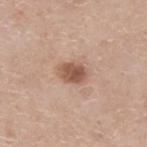{"biopsy_status": "not biopsied; imaged during a skin examination", "image": {"source": "total-body photography crop", "field_of_view_mm": 15}, "patient": {"sex": "female", "age_approx": 75}, "site": "right lower leg", "automated_metrics": {"area_mm2_approx": 6.0, "eccentricity": 0.7, "shape_asymmetry": 0.15}, "lighting": "white-light"}